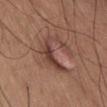{"patient": {"sex": "male", "age_approx": 65}, "automated_metrics": {"lesion_detection_confidence_0_100": 70}, "image": {"source": "total-body photography crop", "field_of_view_mm": 15}, "site": "abdomen"}